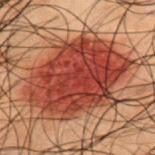{
  "automated_metrics": {
    "border_irregularity_0_10": 2.5,
    "color_variation_0_10": 4.5,
    "nevus_likeness_0_100": 100,
    "lesion_detection_confidence_0_100": 100
  },
  "lesion_size": {
    "long_diameter_mm_approx": 10.0
  },
  "site": "upper back",
  "image": {
    "source": "total-body photography crop",
    "field_of_view_mm": 15
  },
  "lighting": "cross-polarized",
  "patient": {
    "sex": "male",
    "age_approx": 50
  }
}